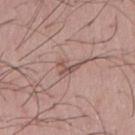The recorded lesion diameter is about 3 mm.
Imaged with white-light lighting.
A male subject, aged 53 to 57.
A lesion tile, about 15 mm wide, cut from a 3D total-body photograph.
On the chest.